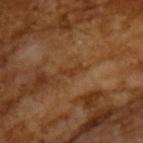Impression:
No biopsy was performed on this lesion — it was imaged during a full skin examination and was not determined to be concerning.
Context:
Cropped from a total-body skin-imaging series; the visible field is about 15 mm. Imaged with cross-polarized lighting. The lesion's longest dimension is about 3 mm. An algorithmic analysis of the crop reported a border-irregularity rating of about 6/10. And it measured a classifier nevus-likeness of about 0/100. A male patient, roughly 65 years of age.